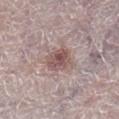The lesion was photographed on a routine skin check and not biopsied; there is no pathology result. Captured under white-light illumination. Approximately 3.5 mm at its widest. A female subject, about 65 years old. On the right lower leg. Automated image analysis of the tile measured roughly 11 lightness units darker than nearby skin and a normalized lesion–skin contrast near 7.5. Cropped from a total-body skin-imaging series; the visible field is about 15 mm.